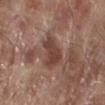Assessment:
Recorded during total-body skin imaging; not selected for excision or biopsy.
Background:
From the right lower leg. The recorded lesion diameter is about 5 mm. Captured under white-light illumination. A 15 mm crop from a total-body photograph taken for skin-cancer surveillance. The patient is a male in their 70s. Automated image analysis of the tile measured a border-irregularity rating of about 4/10 and internal color variation of about 2 on a 0–10 scale. The analysis additionally found a classifier nevus-likeness of about 0/100 and a detector confidence of about 90 out of 100 that the crop contains a lesion.